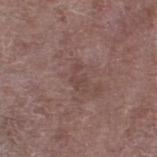Recorded during total-body skin imaging; not selected for excision or biopsy. Longest diameter approximately 2.5 mm. A male subject, about 70 years old. Captured under white-light illumination. The total-body-photography lesion software estimated a lesion area of about 3 mm². The analysis additionally found border irregularity of about 7 on a 0–10 scale, a color-variation rating of about 0/10, and a peripheral color-asymmetry measure near 0. It also reported a nevus-likeness score of about 0/100 and a lesion-detection confidence of about 100/100. Located on the leg. A 15 mm crop from a total-body photograph taken for skin-cancer surveillance.